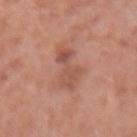Captured during whole-body skin photography for melanoma surveillance; the lesion was not biopsied. A 15 mm close-up extracted from a 3D total-body photography capture. The tile uses white-light illumination. Approximately 5.5 mm at its widest. The lesion is located on the right forearm. Automated tile analysis of the lesion measured an outline eccentricity of about 0.95 (0 = round, 1 = elongated). The software also gave a border-irregularity rating of about 6/10, a within-lesion color-variation index near 3.5/10, and peripheral color asymmetry of about 1. It also reported a classifier nevus-likeness of about 0/100 and lesion-presence confidence of about 100/100. A female subject aged approximately 40.